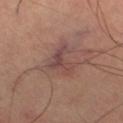biopsy status = imaged on a skin check; not biopsied
lighting = cross-polarized
imaging modality = ~15 mm tile from a whole-body skin photo
patient = male, aged 63 to 67
location = the right thigh
diameter = ~4.5 mm (longest diameter)